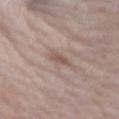Q: Was this lesion biopsied?
A: total-body-photography surveillance lesion; no biopsy
Q: Illumination type?
A: white-light
Q: Automated lesion metrics?
A: about 9 CIELAB-L* units darker than the surrounding skin and a normalized lesion–skin contrast near 6.5; border irregularity of about 4 on a 0–10 scale, a within-lesion color-variation index near 0/10, and radial color variation of about 0
Q: What is the lesion's diameter?
A: ~2.5 mm (longest diameter)
Q: Who is the patient?
A: female, aged 68 to 72
Q: What kind of image is this?
A: ~15 mm crop, total-body skin-cancer survey
Q: What is the anatomic site?
A: the right upper arm